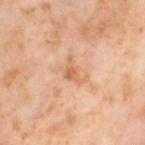<lesion>
  <biopsy_status>not biopsied; imaged during a skin examination</biopsy_status>
  <lighting>cross-polarized</lighting>
  <patient>
    <sex>female</sex>
    <age_approx>55</age_approx>
  </patient>
  <site>right thigh</site>
  <image>
    <source>total-body photography crop</source>
    <field_of_view_mm>15</field_of_view_mm>
  </image>
</lesion>